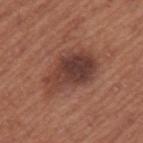{"biopsy_status": "not biopsied; imaged during a skin examination", "patient": {"sex": "female", "age_approx": 65}, "lesion_size": {"long_diameter_mm_approx": 6.5}, "image": {"source": "total-body photography crop", "field_of_view_mm": 15}, "site": "left upper arm"}